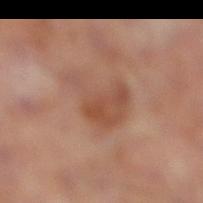The lesion was photographed on a routine skin check and not biopsied; there is no pathology result. Cropped from a whole-body photographic skin survey; the tile spans about 15 mm. Automated tile analysis of the lesion measured a lesion area of about 15 mm², an outline eccentricity of about 0.6 (0 = round, 1 = elongated), and a shape-asymmetry score of about 0.5 (0 = symmetric). The lesion is located on the right lower leg. A male subject aged around 65.